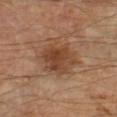Imaged during a routine full-body skin examination; the lesion was not biopsied and no histopathology is available. A 15 mm close-up tile from a total-body photography series done for melanoma screening. The patient is a male aged approximately 70. Located on the left forearm. Captured under cross-polarized illumination.